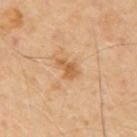automated lesion analysis — an outline eccentricity of about 0.8 (0 = round, 1 = elongated) and a symmetry-axis asymmetry near 0.35; border irregularity of about 4 on a 0–10 scale, internal color variation of about 2 on a 0–10 scale, and a peripheral color-asymmetry measure near 0.5; a nevus-likeness score of about 0/100 and a lesion-detection confidence of about 100/100 | image — total-body-photography crop, ~15 mm field of view | location — the mid back | subject — male, in their 70s | lesion size — ≈3 mm | illumination — cross-polarized illumination.